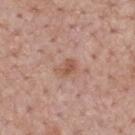follow-up: no biopsy performed (imaged during a skin exam) | size: about 2.5 mm | subject: male, aged 53 to 57 | automated lesion analysis: a lesion color around L≈55 a*≈22 b*≈29 in CIELAB, a lesion–skin lightness drop of about 8, and a normalized lesion–skin contrast near 6.5; border irregularity of about 2.5 on a 0–10 scale, a within-lesion color-variation index near 2.5/10, and a peripheral color-asymmetry measure near 1; an automated nevus-likeness rating near 0 out of 100 and a detector confidence of about 100 out of 100 that the crop contains a lesion | image source: total-body-photography crop, ~15 mm field of view | location: the mid back.